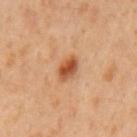Part of a total-body skin-imaging series; this lesion was reviewed on a skin check and was not flagged for biopsy.
This image is a 15 mm lesion crop taken from a total-body photograph.
The lesion is on the mid back.
A male subject, in their 60s.